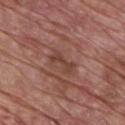  biopsy_status: not biopsied; imaged during a skin examination
  site: chest
  patient:
    sex: male
    age_approx: 65
  lighting: white-light
  image:
    source: total-body photography crop
    field_of_view_mm: 15
  lesion_size:
    long_diameter_mm_approx: 3.0